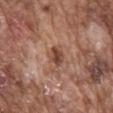Impression:
The lesion was photographed on a routine skin check and not biopsied; there is no pathology result.
Acquisition and patient details:
The lesion-visualizer software estimated a lesion area of about 4 mm², a shape eccentricity near 0.5, and two-axis asymmetry of about 0.2. The analysis additionally found a lesion color around L≈45 a*≈22 b*≈28 in CIELAB, about 11 CIELAB-L* units darker than the surrounding skin, and a normalized border contrast of about 8.5. And it measured border irregularity of about 2 on a 0–10 scale, a color-variation rating of about 4/10, and peripheral color asymmetry of about 1.5. It also reported a nevus-likeness score of about 50/100. The lesion is located on the back. Measured at roughly 2.5 mm in maximum diameter. This is a white-light tile. A 15 mm close-up tile from a total-body photography series done for melanoma screening. A male patient aged 73 to 77.